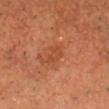{
  "biopsy_status": "not biopsied; imaged during a skin examination",
  "site": "head or neck",
  "patient": {
    "sex": "male",
    "age_approx": 65
  },
  "lighting": "cross-polarized",
  "image": {
    "source": "total-body photography crop",
    "field_of_view_mm": 15
  },
  "automated_metrics": {
    "area_mm2_approx": 4.5,
    "eccentricity": 0.75,
    "shape_asymmetry": 0.3,
    "cielab_L": 46,
    "cielab_a": 28,
    "cielab_b": 37,
    "vs_skin_darker_L": 6.0,
    "vs_skin_contrast_norm": 5.0,
    "border_irregularity_0_10": 3.5,
    "color_variation_0_10": 1.5,
    "peripheral_color_asymmetry": 0.5,
    "nevus_likeness_0_100": 0,
    "lesion_detection_confidence_0_100": 100
  },
  "lesion_size": {
    "long_diameter_mm_approx": 2.5
  }
}